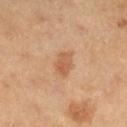This lesion was catalogued during total-body skin photography and was not selected for biopsy.
This is a cross-polarized tile.
A female subject aged around 60.
This image is a 15 mm lesion crop taken from a total-body photograph.
On the right lower leg.